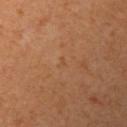The lesion was photographed on a routine skin check and not biopsied; there is no pathology result. A lesion tile, about 15 mm wide, cut from a 3D total-body photograph. The lesion is on the left upper arm. A female patient, aged 53 to 57. The tile uses cross-polarized illumination. Longest diameter approximately 1 mm.